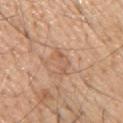The lesion was photographed on a routine skin check and not biopsied; there is no pathology result.
The tile uses white-light illumination.
A 15 mm close-up tile from a total-body photography series done for melanoma screening.
The lesion's longest dimension is about 3.5 mm.
From the upper back.
The lesion-visualizer software estimated a lesion area of about 4 mm², an eccentricity of roughly 0.85, and a symmetry-axis asymmetry near 0.55.
A male subject, approximately 55 years of age.